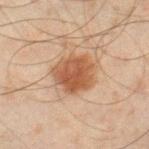biopsy status: no biopsy performed (imaged during a skin exam) | anatomic site: the right thigh | lighting: cross-polarized illumination | lesion diameter: about 4.5 mm | image source: total-body-photography crop, ~15 mm field of view | image-analysis metrics: an outline eccentricity of about 0.35 (0 = round, 1 = elongated) and two-axis asymmetry of about 0.2; a border-irregularity rating of about 2/10, a within-lesion color-variation index near 3.5/10, and peripheral color asymmetry of about 1; a classifier nevus-likeness of about 100/100 and lesion-presence confidence of about 100/100 | subject: male, aged around 45.